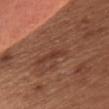Q: Is there a histopathology result?
A: no biopsy performed (imaged during a skin exam)
Q: How was this image acquired?
A: total-body-photography crop, ~15 mm field of view
Q: Patient demographics?
A: female, aged 63 to 67
Q: Lesion size?
A: ~3 mm (longest diameter)
Q: Automated lesion metrics?
A: a lesion area of about 2 mm², an outline eccentricity of about 0.95 (0 = round, 1 = elongated), and a symmetry-axis asymmetry near 0.35; a lesion–skin lightness drop of about 7 and a lesion-to-skin contrast of about 6 (normalized; higher = more distinct); a border-irregularity index near 4/10 and a color-variation rating of about 0/10; an automated nevus-likeness rating near 0 out of 100 and a lesion-detection confidence of about 55/100
Q: How was the tile lit?
A: white-light illumination
Q: Where on the body is the lesion?
A: the chest